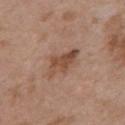Q: Was this lesion biopsied?
A: catalogued during a skin exam; not biopsied
Q: What did automated image analysis measure?
A: an outline eccentricity of about 0.85 (0 = round, 1 = elongated) and two-axis asymmetry of about 0.35; a lesion color around L≈48 a*≈20 b*≈29 in CIELAB and roughly 10 lightness units darker than nearby skin; a classifier nevus-likeness of about 40/100 and lesion-presence confidence of about 100/100
Q: Who is the patient?
A: female, in their 30s
Q: Lesion location?
A: the upper back
Q: What is the imaging modality?
A: total-body-photography crop, ~15 mm field of view